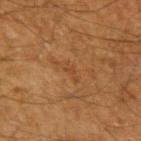Imaged during a routine full-body skin examination; the lesion was not biopsied and no histopathology is available.
The subject is a male aged around 60.
Automated image analysis of the tile measured a lesion color around L≈40 a*≈22 b*≈34 in CIELAB and a lesion–skin lightness drop of about 5. The software also gave a border-irregularity index near 4/10 and peripheral color asymmetry of about 0.
A 15 mm crop from a total-body photograph taken for skin-cancer surveillance.
Located on the arm.
Measured at roughly 1 mm in maximum diameter.